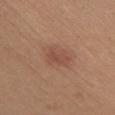{
  "biopsy_status": "not biopsied; imaged during a skin examination",
  "image": {
    "source": "total-body photography crop",
    "field_of_view_mm": 15
  },
  "patient": {
    "sex": "female",
    "age_approx": 45
  },
  "site": "chest"
}